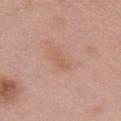| feature | finding |
|---|---|
| biopsy status | imaged on a skin check; not biopsied |
| anatomic site | the chest |
| patient | female, in their mid-60s |
| lighting | white-light illumination |
| lesion diameter | about 2.5 mm |
| imaging modality | ~15 mm crop, total-body skin-cancer survey |